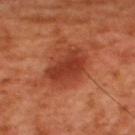notes = no biopsy performed (imaged during a skin exam); acquisition = ~15 mm tile from a whole-body skin photo; subject = male, approximately 50 years of age; lighting = cross-polarized illumination; body site = the upper back.